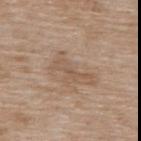{
  "biopsy_status": "not biopsied; imaged during a skin examination",
  "image": {
    "source": "total-body photography crop",
    "field_of_view_mm": 15
  },
  "site": "back",
  "patient": {
    "sex": "female",
    "age_approx": 75
  },
  "lesion_size": {
    "long_diameter_mm_approx": 4.5
  }
}